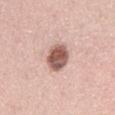Captured during whole-body skin photography for melanoma surveillance; the lesion was not biopsied.
The patient is a female approximately 40 years of age.
The lesion is located on the back.
The lesion-visualizer software estimated a footprint of about 9 mm² and an eccentricity of roughly 0.7. The software also gave a lesion color around L≈56 a*≈21 b*≈24 in CIELAB, about 19 CIELAB-L* units darker than the surrounding skin, and a normalized border contrast of about 11.5. And it measured a border-irregularity index near 1/10 and peripheral color asymmetry of about 2. It also reported a classifier nevus-likeness of about 60/100 and a lesion-detection confidence of about 100/100.
A lesion tile, about 15 mm wide, cut from a 3D total-body photograph.